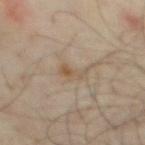The lesion was photographed on a routine skin check and not biopsied; there is no pathology result. Located on the chest. A 15 mm crop from a total-body photograph taken for skin-cancer surveillance. The patient is a male in their mid-60s. The total-body-photography lesion software estimated an area of roughly 4 mm², a shape eccentricity near 0.85, and a symmetry-axis asymmetry near 0.4. The analysis additionally found a mean CIELAB color near L≈51 a*≈13 b*≈28, roughly 7 lightness units darker than nearby skin, and a lesion-to-skin contrast of about 6 (normalized; higher = more distinct). This is a cross-polarized tile.